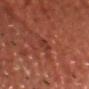biopsy status=catalogued during a skin exam; not biopsied | diameter=≈3 mm | illumination=cross-polarized illumination | patient=male, approximately 45 years of age | acquisition=15 mm crop, total-body photography | body site=the front of the torso.